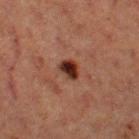Assessment: This lesion was catalogued during total-body skin photography and was not selected for biopsy. Context: Cropped from a whole-body photographic skin survey; the tile spans about 15 mm. The lesion is on the left thigh. A female subject aged 38–42.